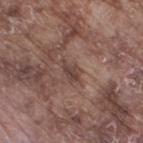This lesion was catalogued during total-body skin photography and was not selected for biopsy. This is a white-light tile. The patient is a male approximately 75 years of age. Located on the right thigh. A 15 mm close-up tile from a total-body photography series done for melanoma screening.